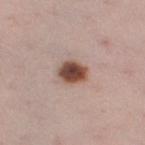Q: Was a biopsy performed?
A: imaged on a skin check; not biopsied
Q: How was the tile lit?
A: white-light
Q: Patient demographics?
A: female, roughly 35 years of age
Q: Where on the body is the lesion?
A: the right thigh
Q: How was this image acquired?
A: total-body-photography crop, ~15 mm field of view
Q: Lesion size?
A: about 3.5 mm